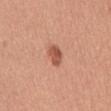The lesion was tiled from a total-body skin photograph and was not biopsied.
A male subject, in their mid- to late 40s.
Located on the mid back.
The lesion's longest dimension is about 3 mm.
Automated tile analysis of the lesion measured an eccentricity of roughly 0.75 and a shape-asymmetry score of about 0.25 (0 = symmetric). The software also gave an average lesion color of about L≈54 a*≈27 b*≈32 (CIELAB), roughly 12 lightness units darker than nearby skin, and a normalized border contrast of about 8.5. And it measured a border-irregularity rating of about 2/10, a within-lesion color-variation index near 2.5/10, and a peripheral color-asymmetry measure near 1.
This image is a 15 mm lesion crop taken from a total-body photograph.